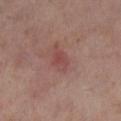Clinical impression:
Captured during whole-body skin photography for melanoma surveillance; the lesion was not biopsied.
Image and clinical context:
This is a cross-polarized tile. On the left lower leg. The lesion's longest dimension is about 3 mm. A 15 mm crop from a total-body photograph taken for skin-cancer surveillance. The total-body-photography lesion software estimated an average lesion color of about L≈45 a*≈24 b*≈22 (CIELAB), about 6 CIELAB-L* units darker than the surrounding skin, and a normalized border contrast of about 5. And it measured a border-irregularity rating of about 1.5/10, internal color variation of about 2.5 on a 0–10 scale, and a peripheral color-asymmetry measure near 1. And it measured a classifier nevus-likeness of about 10/100 and a detector confidence of about 100 out of 100 that the crop contains a lesion. The patient is a female about 40 years old.